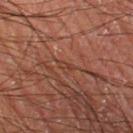The tile uses cross-polarized illumination. Approximately 2 mm at its widest. A male subject, aged approximately 55. On the left thigh. Cropped from a whole-body photographic skin survey; the tile spans about 15 mm. An algorithmic analysis of the crop reported a lesion color around L≈35 a*≈21 b*≈26 in CIELAB, a lesion–skin lightness drop of about 4, and a normalized lesion–skin contrast near 4.5. And it measured a border-irregularity rating of about 3/10, a within-lesion color-variation index near 0/10, and a peripheral color-asymmetry measure near 0. It also reported a nevus-likeness score of about 0/100 and a lesion-detection confidence of about 0/100.